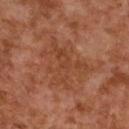Q: Is there a histopathology result?
A: catalogued during a skin exam; not biopsied
Q: What is the imaging modality?
A: ~15 mm tile from a whole-body skin photo
Q: Who is the patient?
A: female, approximately 60 years of age
Q: Lesion location?
A: the back